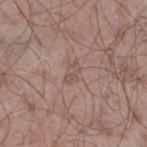No biopsy was performed on this lesion — it was imaged during a full skin examination and was not determined to be concerning.
A male subject, about 55 years old.
The lesion is on the leg.
A roughly 15 mm field-of-view crop from a total-body skin photograph.
Approximately 2.5 mm at its widest.